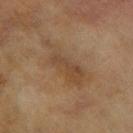Imaged during a routine full-body skin examination; the lesion was not biopsied and no histopathology is available.
A female patient aged around 60.
An algorithmic analysis of the crop reported an area of roughly 14 mm², an eccentricity of roughly 0.9, and two-axis asymmetry of about 0.3. The software also gave an average lesion color of about L≈41 a*≈15 b*≈29 (CIELAB), roughly 6 lightness units darker than nearby skin, and a normalized border contrast of about 5.5. And it measured border irregularity of about 5 on a 0–10 scale and peripheral color asymmetry of about 1.5.
The lesion is located on the right forearm.
This is a cross-polarized tile.
A lesion tile, about 15 mm wide, cut from a 3D total-body photograph.
Measured at roughly 7.5 mm in maximum diameter.